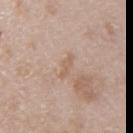| key | value |
|---|---|
| notes | imaged on a skin check; not biopsied |